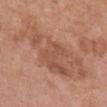<lesion>
<biopsy_status>not biopsied; imaged during a skin examination</biopsy_status>
<automated_metrics>
  <area_mm2_approx>38.0</area_mm2_approx>
  <eccentricity>0.9</eccentricity>
  <border_irregularity_0_10>6.0</border_irregularity_0_10>
  <color_variation_0_10>4.5</color_variation_0_10>
  <peripheral_color_asymmetry>1.5</peripheral_color_asymmetry>
  <nevus_likeness_0_100>0</nevus_likeness_0_100>
  <lesion_detection_confidence_0_100>100</lesion_detection_confidence_0_100>
</automated_metrics>
<image>
  <source>total-body photography crop</source>
  <field_of_view_mm>15</field_of_view_mm>
</image>
<lighting>white-light</lighting>
<site>chest</site>
<lesion_size>
  <long_diameter_mm_approx>10.5</long_diameter_mm_approx>
</lesion_size>
<patient>
  <sex>female</sex>
  <age_approx>65</age_approx>
</patient>
</lesion>